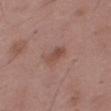workup: catalogued during a skin exam; not biopsied
anatomic site: the right thigh
image-analysis metrics: a lesion area of about 5 mm² and a shape-asymmetry score of about 0.25 (0 = symmetric); a border-irregularity rating of about 2.5/10, internal color variation of about 3.5 on a 0–10 scale, and a peripheral color-asymmetry measure near 1; a nevus-likeness score of about 25/100 and lesion-presence confidence of about 100/100
image: 15 mm crop, total-body photography
lesion diameter: ~3.5 mm (longest diameter)
subject: male, aged around 50